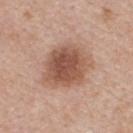Captured during whole-body skin photography for melanoma surveillance; the lesion was not biopsied.